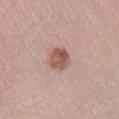Notes:
* notes — total-body-photography surveillance lesion; no biopsy